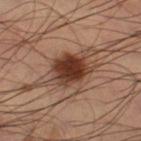Clinical impression: Recorded during total-body skin imaging; not selected for excision or biopsy. Context: A male subject aged approximately 60. This image is a 15 mm lesion crop taken from a total-body photograph. Automated tile analysis of the lesion measured an average lesion color of about L≈41 a*≈19 b*≈27 (CIELAB) and a lesion-to-skin contrast of about 9.5 (normalized; higher = more distinct). And it measured an automated nevus-likeness rating near 100 out of 100. Longest diameter approximately 11 mm. The lesion is located on the leg.